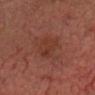Q: Was a biopsy performed?
A: no biopsy performed (imaged during a skin exam)
Q: Patient demographics?
A: male, roughly 75 years of age
Q: What is the imaging modality?
A: total-body-photography crop, ~15 mm field of view
Q: How was the tile lit?
A: cross-polarized illumination
Q: Lesion location?
A: the head or neck